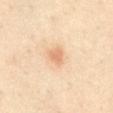{
  "biopsy_status": "not biopsied; imaged during a skin examination",
  "site": "abdomen",
  "patient": {
    "sex": "female",
    "age_approx": 40
  },
  "automated_metrics": {
    "cielab_L": 71,
    "cielab_a": 22,
    "cielab_b": 36,
    "vs_skin_darker_L": 9.0,
    "vs_skin_contrast_norm": 5.5,
    "border_irregularity_0_10": 2.5,
    "color_variation_0_10": 2.0,
    "peripheral_color_asymmetry": 1.0
  },
  "image": {
    "source": "total-body photography crop",
    "field_of_view_mm": 15
  },
  "lesion_size": {
    "long_diameter_mm_approx": 2.0
  },
  "lighting": "cross-polarized"
}